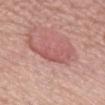follow-up: catalogued during a skin exam; not biopsied
image: 15 mm crop, total-body photography
subject: female, in their mid-60s
location: the back
automated metrics: an outline eccentricity of about 0.9 (0 = round, 1 = elongated); a border-irregularity index near 7/10, a color-variation rating of about 2.5/10, and a peripheral color-asymmetry measure near 0.5; a lesion-detection confidence of about 70/100
lighting: white-light illumination